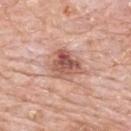Clinical impression:
This lesion was catalogued during total-body skin photography and was not selected for biopsy.
Context:
Cropped from a total-body skin-imaging series; the visible field is about 15 mm. This is a white-light tile. Located on the upper back. A male patient approximately 80 years of age.